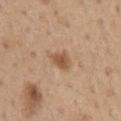Context: A male patient aged approximately 65. The lesion is on the front of the torso. Imaged with white-light lighting. About 3 mm across. A 15 mm close-up tile from a total-body photography series done for melanoma screening. The lesion-visualizer software estimated a border-irregularity index near 2.5/10, internal color variation of about 2.5 on a 0–10 scale, and radial color variation of about 0.5. And it measured a classifier nevus-likeness of about 70/100.